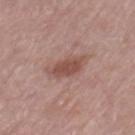follow-up = no biopsy performed (imaged during a skin exam); acquisition = ~15 mm crop, total-body skin-cancer survey; anatomic site = the left thigh; subject = female, about 65 years old.